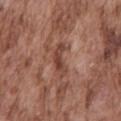  biopsy_status: not biopsied; imaged during a skin examination
  patient:
    sex: male
    age_approx: 75
  image:
    source: total-body photography crop
    field_of_view_mm: 15
  site: mid back